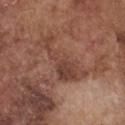Impression:
Recorded during total-body skin imaging; not selected for excision or biopsy.
Context:
Longest diameter approximately 7 mm. A male subject aged around 75. On the chest. A close-up tile cropped from a whole-body skin photograph, about 15 mm across.